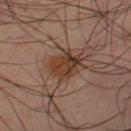Q: Was a biopsy performed?
A: no biopsy performed (imaged during a skin exam)
Q: What kind of image is this?
A: 15 mm crop, total-body photography
Q: What are the patient's age and sex?
A: male, aged approximately 40
Q: Where on the body is the lesion?
A: the left lower leg
Q: How was the tile lit?
A: cross-polarized illumination
Q: Lesion size?
A: about 3.5 mm
Q: Automated lesion metrics?
A: a border-irregularity index near 2.5/10 and internal color variation of about 5 on a 0–10 scale; a classifier nevus-likeness of about 35/100